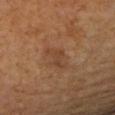Captured during whole-body skin photography for melanoma surveillance; the lesion was not biopsied. The lesion is on the arm. The lesion's longest dimension is about 4 mm. A female subject, aged 58–62. This is a cross-polarized tile. This image is a 15 mm lesion crop taken from a total-body photograph.